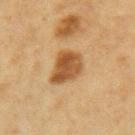No biopsy was performed on this lesion — it was imaged during a full skin examination and was not determined to be concerning. A female patient, in their mid-40s. An algorithmic analysis of the crop reported an average lesion color of about L≈46 a*≈19 b*≈36 (CIELAB) and about 12 CIELAB-L* units darker than the surrounding skin. The software also gave border irregularity of about 2.5 on a 0–10 scale and radial color variation of about 1.5. On the right forearm. A 15 mm close-up extracted from a 3D total-body photography capture. About 3.5 mm across.